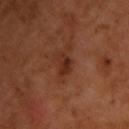Assessment: This lesion was catalogued during total-body skin photography and was not selected for biopsy.